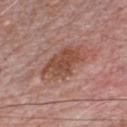biopsy_status: not biopsied; imaged during a skin examination
site: chest
lighting: white-light
image:
  source: total-body photography crop
  field_of_view_mm: 15
patient:
  sex: male
  age_approx: 70
lesion_size:
  long_diameter_mm_approx: 6.0